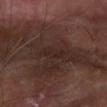Assessment:
Captured during whole-body skin photography for melanoma surveillance; the lesion was not biopsied.
Image and clinical context:
Automated image analysis of the tile measured an area of roughly 4.5 mm², an outline eccentricity of about 0.9 (0 = round, 1 = elongated), and a symmetry-axis asymmetry near 0.15. The analysis additionally found a lesion color around L≈23 a*≈15 b*≈18 in CIELAB and about 4 CIELAB-L* units darker than the surrounding skin. And it measured border irregularity of about 2.5 on a 0–10 scale, internal color variation of about 1 on a 0–10 scale, and a peripheral color-asymmetry measure near 0.5. The analysis additionally found a classifier nevus-likeness of about 0/100 and lesion-presence confidence of about 80/100. Captured under cross-polarized illumination. Longest diameter approximately 3 mm. A 15 mm close-up tile from a total-body photography series done for melanoma screening. The lesion is on the left forearm. A male patient, aged around 70.